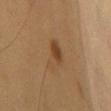Q: Was this lesion biopsied?
A: no biopsy performed (imaged during a skin exam)
Q: Patient demographics?
A: male, approximately 55 years of age
Q: Lesion location?
A: the chest
Q: Lesion size?
A: ~4.5 mm (longest diameter)
Q: How was this image acquired?
A: 15 mm crop, total-body photography